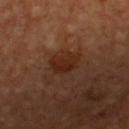lighting: cross-polarized illumination; lesion size: ~3.5 mm (longest diameter); subject: male, in their 50s; location: the chest; imaging modality: ~15 mm tile from a whole-body skin photo.